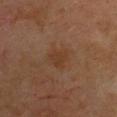Image and clinical context: A 15 mm close-up extracted from a 3D total-body photography capture. From the chest. A female subject in their mid- to late 50s. The recorded lesion diameter is about 2.5 mm. Imaged with cross-polarized lighting.